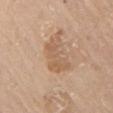The lesion was tiled from a total-body skin photograph and was not biopsied.
Captured under white-light illumination.
The lesion-visualizer software estimated a lesion area of about 9.5 mm², a shape eccentricity near 0.75, and a symmetry-axis asymmetry near 0.4. It also reported a lesion color around L≈59 a*≈18 b*≈33 in CIELAB, about 8 CIELAB-L* units darker than the surrounding skin, and a normalized lesion–skin contrast near 5.5. The analysis additionally found a classifier nevus-likeness of about 0/100 and a lesion-detection confidence of about 100/100.
A male patient in their 80s.
The lesion's longest dimension is about 4.5 mm.
A 15 mm close-up extracted from a 3D total-body photography capture.
On the right upper arm.